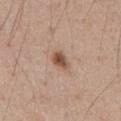Notes:
• follow-up — no biopsy performed (imaged during a skin exam)
• site — the abdomen
• automated lesion analysis — an area of roughly 4 mm², an outline eccentricity of about 0.7 (0 = round, 1 = elongated), and two-axis asymmetry of about 0.25; a lesion–skin lightness drop of about 13; border irregularity of about 2 on a 0–10 scale, internal color variation of about 4 on a 0–10 scale, and a peripheral color-asymmetry measure near 1; a nevus-likeness score of about 95/100 and a lesion-detection confidence of about 100/100
• lesion diameter — about 2.5 mm
• patient — male, in their 60s
• acquisition — ~15 mm crop, total-body skin-cancer survey